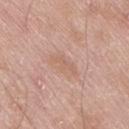The lesion is located on the leg. The patient is a male roughly 80 years of age. A region of skin cropped from a whole-body photographic capture, roughly 15 mm wide. An algorithmic analysis of the crop reported a footprint of about 3.5 mm². And it measured a lesion color around L≈61 a*≈20 b*≈29 in CIELAB, about 6 CIELAB-L* units darker than the surrounding skin, and a normalized border contrast of about 4.5. The software also gave an automated nevus-likeness rating near 0 out of 100 and a lesion-detection confidence of about 100/100. Approximately 3.5 mm at its widest.